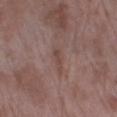workup: no biopsy performed (imaged during a skin exam); image source: ~15 mm crop, total-body skin-cancer survey; patient: female, aged around 70; body site: the left lower leg; diameter: ≈3 mm; illumination: white-light illumination.